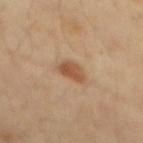This lesion was catalogued during total-body skin photography and was not selected for biopsy.
The lesion is located on the back.
A region of skin cropped from a whole-body photographic capture, roughly 15 mm wide.
A male patient, aged approximately 30.